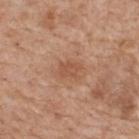{
  "image": {
    "source": "total-body photography crop",
    "field_of_view_mm": 15
  },
  "patient": {
    "sex": "male",
    "age_approx": 60
  },
  "site": "upper back",
  "automated_metrics": {
    "vs_skin_contrast_norm": 5.5,
    "nevus_likeness_0_100": 0,
    "lesion_detection_confidence_0_100": 100
  },
  "lesion_size": {
    "long_diameter_mm_approx": 3.0
  }
}